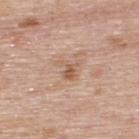Q: Is there a histopathology result?
A: no biopsy performed (imaged during a skin exam)
Q: What is the imaging modality?
A: ~15 mm tile from a whole-body skin photo
Q: Lesion size?
A: about 3 mm
Q: Where on the body is the lesion?
A: the upper back
Q: What are the patient's age and sex?
A: male, aged around 75
Q: Automated lesion metrics?
A: an average lesion color of about L≈58 a*≈20 b*≈31 (CIELAB), roughly 9 lightness units darker than nearby skin, and a normalized lesion–skin contrast near 6.5; a border-irregularity index near 6/10, a color-variation rating of about 2/10, and radial color variation of about 0.5; a nevus-likeness score of about 0/100 and lesion-presence confidence of about 100/100